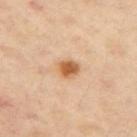Captured during whole-body skin photography for melanoma surveillance; the lesion was not biopsied. The lesion-visualizer software estimated about 14 CIELAB-L* units darker than the surrounding skin and a normalized lesion–skin contrast near 9.5. From the right upper arm. A 15 mm close-up tile from a total-body photography series done for melanoma screening. The subject is a female aged approximately 45.